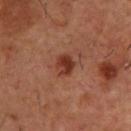No biopsy was performed on this lesion — it was imaged during a full skin examination and was not determined to be concerning.
Captured under cross-polarized illumination.
A male patient, aged 53–57.
An algorithmic analysis of the crop reported border irregularity of about 2 on a 0–10 scale and a peripheral color-asymmetry measure near 1.5. It also reported a detector confidence of about 100 out of 100 that the crop contains a lesion.
About 3 mm across.
A lesion tile, about 15 mm wide, cut from a 3D total-body photograph.
The lesion is on the upper back.